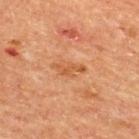biopsy_status: not biopsied; imaged during a skin examination
lighting: cross-polarized
image:
  source: total-body photography crop
  field_of_view_mm: 15
patient:
  sex: male
  age_approx: 65
site: back
lesion_size:
  long_diameter_mm_approx: 3.5